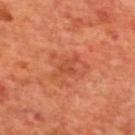Captured during whole-body skin photography for melanoma surveillance; the lesion was not biopsied.
Automated tile analysis of the lesion measured a lesion area of about 4 mm² and an eccentricity of roughly 0.85. The analysis additionally found a mean CIELAB color near L≈49 a*≈32 b*≈37, about 7 CIELAB-L* units darker than the surrounding skin, and a normalized border contrast of about 5. And it measured a nevus-likeness score of about 0/100 and a detector confidence of about 100 out of 100 that the crop contains a lesion.
The lesion is located on the mid back.
Captured under cross-polarized illumination.
A male subject in their 70s.
The recorded lesion diameter is about 3.5 mm.
A 15 mm close-up extracted from a 3D total-body photography capture.